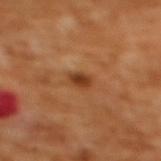– workup — total-body-photography surveillance lesion; no biopsy
– lesion diameter — ~2.5 mm (longest diameter)
– TBP lesion metrics — a footprint of about 3.5 mm², an outline eccentricity of about 0.7 (0 = round, 1 = elongated), and two-axis asymmetry of about 0.2
– subject — female, aged approximately 55
– tile lighting — cross-polarized
– image source — total-body-photography crop, ~15 mm field of view
– body site — the back